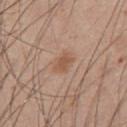{
  "biopsy_status": "not biopsied; imaged during a skin examination"
}